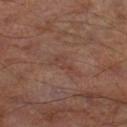– biopsy status: total-body-photography surveillance lesion; no biopsy
– imaging modality: 15 mm crop, total-body photography
– lesion size: about 3 mm
– anatomic site: the leg
– subject: male, aged approximately 65
– lighting: cross-polarized illumination